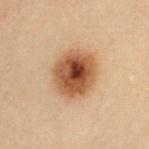{"site": "chest", "image": {"source": "total-body photography crop", "field_of_view_mm": 15}, "patient": {"sex": "female", "age_approx": 20}}